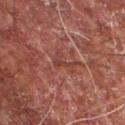Q: How large is the lesion?
A: ~3 mm (longest diameter)
Q: Who is the patient?
A: male, roughly 55 years of age
Q: What kind of image is this?
A: total-body-photography crop, ~15 mm field of view
Q: Illumination type?
A: cross-polarized
Q: Automated lesion metrics?
A: an average lesion color of about L≈36 a*≈23 b*≈23 (CIELAB), roughly 6 lightness units darker than nearby skin, and a normalized lesion–skin contrast near 5.5; a border-irregularity rating of about 7.5/10, a color-variation rating of about 0/10, and radial color variation of about 0
Q: Where on the body is the lesion?
A: the front of the torso